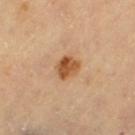Imaged during a routine full-body skin examination; the lesion was not biopsied and no histopathology is available. The lesion is on the left thigh. A female patient, aged around 70. A 15 mm close-up extracted from a 3D total-body photography capture.